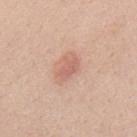Captured during whole-body skin photography for melanoma surveillance; the lesion was not biopsied.
The lesion-visualizer software estimated an average lesion color of about L≈62 a*≈24 b*≈28 (CIELAB) and about 9 CIELAB-L* units darker than the surrounding skin. It also reported border irregularity of about 3 on a 0–10 scale and a within-lesion color-variation index near 1/10. The software also gave an automated nevus-likeness rating near 80 out of 100 and a detector confidence of about 100 out of 100 that the crop contains a lesion.
Cropped from a total-body skin-imaging series; the visible field is about 15 mm.
The patient is a male approximately 40 years of age.
The lesion is on the upper back.